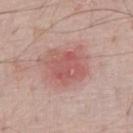Recorded during total-body skin imaging; not selected for excision or biopsy.
This is a white-light tile.
Located on the chest.
A 15 mm crop from a total-body photograph taken for skin-cancer surveillance.
A male subject, in their 70s.
The total-body-photography lesion software estimated a border-irregularity rating of about 2.5/10 and internal color variation of about 4 on a 0–10 scale.
The recorded lesion diameter is about 5.5 mm.